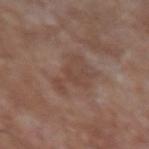Part of a total-body skin-imaging series; this lesion was reviewed on a skin check and was not flagged for biopsy. Measured at roughly 5.5 mm in maximum diameter. An algorithmic analysis of the crop reported an area of roughly 13 mm², an outline eccentricity of about 0.8 (0 = round, 1 = elongated), and a symmetry-axis asymmetry near 0.5. And it measured a border-irregularity index near 6.5/10, a color-variation rating of about 2.5/10, and peripheral color asymmetry of about 0.5. It also reported an automated nevus-likeness rating near 0 out of 100 and a detector confidence of about 100 out of 100 that the crop contains a lesion. This is a white-light tile. On the left upper arm. The subject is a male in their mid- to late 60s. Cropped from a whole-body photographic skin survey; the tile spans about 15 mm.